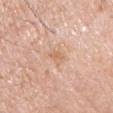Q: Was this lesion biopsied?
A: imaged on a skin check; not biopsied
Q: How was this image acquired?
A: ~15 mm crop, total-body skin-cancer survey
Q: Automated lesion metrics?
A: an automated nevus-likeness rating near 0 out of 100 and a detector confidence of about 100 out of 100 that the crop contains a lesion
Q: Lesion location?
A: the chest
Q: How was the tile lit?
A: white-light illumination
Q: Patient demographics?
A: male, aged approximately 60
Q: What is the lesion's diameter?
A: about 2.5 mm